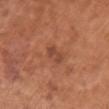A 15 mm close-up tile from a total-body photography series done for melanoma screening. The lesion is on the chest. A female patient, aged around 65.